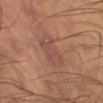Part of a total-body skin-imaging series; this lesion was reviewed on a skin check and was not flagged for biopsy.
The lesion's longest dimension is about 4 mm.
Automated image analysis of the tile measured a footprint of about 9.5 mm² and a shape-asymmetry score of about 0.2 (0 = symmetric). The software also gave a mean CIELAB color near L≈48 a*≈22 b*≈25, a lesion–skin lightness drop of about 6, and a lesion-to-skin contrast of about 5 (normalized; higher = more distinct). And it measured an automated nevus-likeness rating near 0 out of 100 and a detector confidence of about 100 out of 100 that the crop contains a lesion.
A male subject, aged around 45.
Located on the left lower leg.
A close-up tile cropped from a whole-body skin photograph, about 15 mm across.